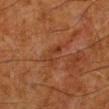No biopsy was performed on this lesion — it was imaged during a full skin examination and was not determined to be concerning. The lesion is on the leg. The lesion's longest dimension is about 3 mm. Imaged with cross-polarized lighting. The patient is a male roughly 80 years of age. A 15 mm close-up extracted from a 3D total-body photography capture.